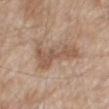{
  "biopsy_status": "not biopsied; imaged during a skin examination",
  "patient": {
    "sex": "male",
    "age_approx": 80
  },
  "site": "mid back",
  "lighting": "white-light",
  "lesion_size": {
    "long_diameter_mm_approx": 6.5
  },
  "automated_metrics": {
    "area_mm2_approx": 16.0,
    "eccentricity": 0.9,
    "shape_asymmetry": 0.45,
    "vs_skin_darker_L": 9.0,
    "border_irregularity_0_10": 6.0,
    "peripheral_color_asymmetry": 1.0,
    "lesion_detection_confidence_0_100": 100
  },
  "image": {
    "source": "total-body photography crop",
    "field_of_view_mm": 15
  }
}